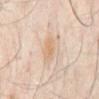– notes: catalogued during a skin exam; not biopsied
– size: ~2.5 mm (longest diameter)
– acquisition: 15 mm crop, total-body photography
– subject: male, aged around 50
– lighting: cross-polarized
– location: the chest